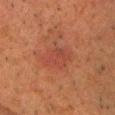Q: Was a biopsy performed?
A: catalogued during a skin exam; not biopsied
Q: Automated lesion metrics?
A: a footprint of about 9 mm² and a shape-asymmetry score of about 0.2 (0 = symmetric); a border-irregularity rating of about 2.5/10 and radial color variation of about 1
Q: Where on the body is the lesion?
A: the head or neck
Q: What lighting was used for the tile?
A: cross-polarized illumination
Q: What kind of image is this?
A: ~15 mm crop, total-body skin-cancer survey
Q: What is the lesion's diameter?
A: ≈4 mm
Q: What are the patient's age and sex?
A: male, aged 63 to 67